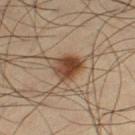anatomic site=the left thigh | patient=male, about 35 years old | image=~15 mm crop, total-body skin-cancer survey | tile lighting=cross-polarized | lesion diameter=about 3.5 mm.